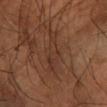No biopsy was performed on this lesion — it was imaged during a full skin examination and was not determined to be concerning.
A male patient, aged around 60.
A close-up tile cropped from a whole-body skin photograph, about 15 mm across.
Located on the arm.
About 6.5 mm across.
Imaged with cross-polarized lighting.